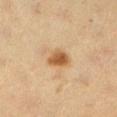workup = total-body-photography surveillance lesion; no biopsy
anatomic site = the left lower leg
patient = female, in their 40s
acquisition = ~15 mm crop, total-body skin-cancer survey